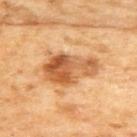Recorded during total-body skin imaging; not selected for excision or biopsy. The patient is a female aged 58 to 62. A lesion tile, about 15 mm wide, cut from a 3D total-body photograph. Longest diameter approximately 6.5 mm. From the upper back. An algorithmic analysis of the crop reported a mean CIELAB color near L≈59 a*≈25 b*≈42 and a normalized border contrast of about 9. The analysis additionally found a border-irregularity rating of about 3.5/10 and a color-variation rating of about 9/10. This is a cross-polarized tile.